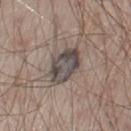| feature | finding |
|---|---|
| workup | catalogued during a skin exam; not biopsied |
| image | 15 mm crop, total-body photography |
| location | the left upper arm |
| patient | male, about 65 years old |